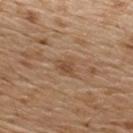The lesion was tiled from a total-body skin photograph and was not biopsied. A male patient about 70 years old. Located on the back. This image is a 15 mm lesion crop taken from a total-body photograph. Captured under white-light illumination. About 2.5 mm across.